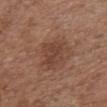biopsy status = no biopsy performed (imaged during a skin exam)
subject = female, aged 73–77
site = the chest
illumination = white-light
TBP lesion metrics = an area of roughly 9.5 mm² and a shape eccentricity near 0.8; a border-irregularity rating of about 3.5/10 and peripheral color asymmetry of about 1
lesion size = ≈4.5 mm
acquisition = total-body-photography crop, ~15 mm field of view